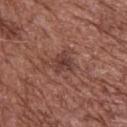  biopsy_status: not biopsied; imaged during a skin examination
  patient:
    sex: male
    age_approx: 75
  automated_metrics:
    border_irregularity_0_10: 3.5
    color_variation_0_10: 3.0
    nevus_likeness_0_100: 30
    lesion_detection_confidence_0_100: 100
  lesion_size:
    long_diameter_mm_approx: 2.5
  image:
    source: total-body photography crop
    field_of_view_mm: 15
  site: upper back
  lighting: white-light